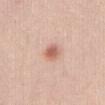{"biopsy_status": "not biopsied; imaged during a skin examination", "image": {"source": "total-body photography crop", "field_of_view_mm": 15}, "site": "abdomen", "patient": {"sex": "female", "age_approx": 25}}